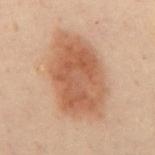* follow-up — no biopsy performed (imaged during a skin exam)
* illumination — cross-polarized
* image — ~15 mm tile from a whole-body skin photo
* TBP lesion metrics — a border-irregularity index near 2/10 and a peripheral color-asymmetry measure near 1; a nevus-likeness score of about 100/100 and a detector confidence of about 100 out of 100 that the crop contains a lesion
* body site — the mid back
* patient — male, about 50 years old